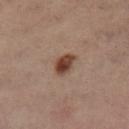biopsy_status: not biopsied; imaged during a skin examination
site: left leg
patient:
  sex: female
  age_approx: 55
lesion_size:
  long_diameter_mm_approx: 2.5
image:
  source: total-body photography crop
  field_of_view_mm: 15